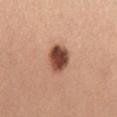– biopsy status — no biopsy performed (imaged during a skin exam)
– lesion diameter — ≈3.5 mm
– subject — female, in their mid- to late 40s
– automated metrics — a shape eccentricity near 0.6 and a shape-asymmetry score of about 0.15 (0 = symmetric)
– lighting — white-light illumination
– imaging modality — ~15 mm crop, total-body skin-cancer survey
– location — the chest